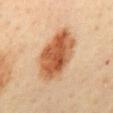  biopsy_status: not biopsied; imaged during a skin examination
  site: mid back
  lesion_size:
    long_diameter_mm_approx: 7.0
  patient:
    sex: female
    age_approx: 45
  lighting: cross-polarized
  image:
    source: total-body photography crop
    field_of_view_mm: 15